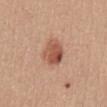The lesion was tiled from a total-body skin photograph and was not biopsied. On the abdomen. Imaged with white-light lighting. A region of skin cropped from a whole-body photographic capture, roughly 15 mm wide. About 3.5 mm across. A female patient approximately 55 years of age.